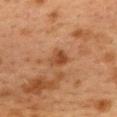Clinical impression: The lesion was photographed on a routine skin check and not biopsied; there is no pathology result. Clinical summary: A female patient about 40 years old. Imaged with cross-polarized lighting. Located on the upper back. A 15 mm close-up extracted from a 3D total-body photography capture.